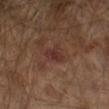Q: Was this lesion biopsied?
A: catalogued during a skin exam; not biopsied
Q: Who is the patient?
A: male, in their mid- to late 60s
Q: Lesion size?
A: about 2.5 mm
Q: What is the anatomic site?
A: the left forearm
Q: What kind of image is this?
A: ~15 mm tile from a whole-body skin photo
Q: What did automated image analysis measure?
A: a lesion area of about 4.5 mm², a shape eccentricity near 0.7, and two-axis asymmetry of about 0.35